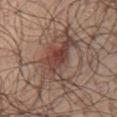No biopsy was performed on this lesion — it was imaged during a full skin examination and was not determined to be concerning.
A male subject, about 65 years old.
From the chest.
Measured at roughly 9 mm in maximum diameter.
A 15 mm crop from a total-body photograph taken for skin-cancer surveillance.